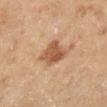Impression:
The lesion was photographed on a routine skin check and not biopsied; there is no pathology result.
Context:
About 3.5 mm across. The lesion is located on the right lower leg. A close-up tile cropped from a whole-body skin photograph, about 15 mm across. A female subject, in their 60s. This is a cross-polarized tile.